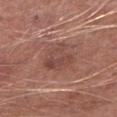Findings:
– size · ≈4 mm
– automated metrics · an area of roughly 11 mm² and a shape eccentricity near 0.45; a nevus-likeness score of about 0/100 and a detector confidence of about 100 out of 100 that the crop contains a lesion
– body site · the left lower leg
– tile lighting · white-light
– acquisition · ~15 mm tile from a whole-body skin photo
– subject · male, roughly 65 years of age